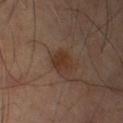Findings:
* follow-up — no biopsy performed (imaged during a skin exam)
* patient — male, roughly 70 years of age
* body site — the left lower leg
* size — ~2.5 mm (longest diameter)
* imaging modality — ~15 mm crop, total-body skin-cancer survey